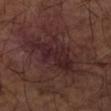The lesion was tiled from a total-body skin photograph and was not biopsied.
The lesion-visualizer software estimated a lesion area of about 16 mm², a shape eccentricity near 0.9, and a symmetry-axis asymmetry near 0.45. It also reported roughly 6 lightness units darker than nearby skin and a normalized lesion–skin contrast near 8. And it measured a border-irregularity rating of about 7/10, a within-lesion color-variation index near 3/10, and peripheral color asymmetry of about 1. The software also gave a nevus-likeness score of about 0/100 and a detector confidence of about 80 out of 100 that the crop contains a lesion.
Measured at roughly 8 mm in maximum diameter.
The tile uses cross-polarized illumination.
The lesion is located on the left forearm.
A 15 mm crop from a total-body photograph taken for skin-cancer surveillance.
A male subject, in their mid- to late 60s.